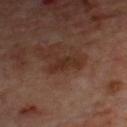Clinical impression:
Imaged during a routine full-body skin examination; the lesion was not biopsied and no histopathology is available.
Clinical summary:
A male subject aged 68–72. The lesion is located on the chest. A roughly 15 mm field-of-view crop from a total-body skin photograph. Imaged with cross-polarized lighting. Approximately 3.5 mm at its widest.